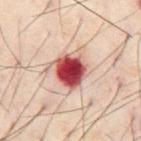notes=no biopsy performed (imaged during a skin exam).